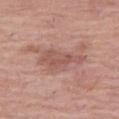Q: Was this lesion biopsied?
A: no biopsy performed (imaged during a skin exam)
Q: Automated lesion metrics?
A: a footprint of about 10 mm², a shape eccentricity near 0.9, and two-axis asymmetry of about 0.4; roughly 9 lightness units darker than nearby skin and a lesion-to-skin contrast of about 6 (normalized; higher = more distinct); border irregularity of about 4.5 on a 0–10 scale and radial color variation of about 0.5
Q: Patient demographics?
A: female, roughly 65 years of age
Q: How was this image acquired?
A: ~15 mm tile from a whole-body skin photo
Q: Illumination type?
A: white-light illumination
Q: Lesion location?
A: the leg
Q: What is the lesion's diameter?
A: about 5.5 mm